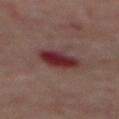Notes:
– workup — imaged on a skin check; not biopsied
– location — the mid back
– imaging modality — ~15 mm tile from a whole-body skin photo
– lesion diameter — about 4.5 mm
– patient — male, in their 70s
– tile lighting — cross-polarized
– TBP lesion metrics — a border-irregularity rating of about 2/10, internal color variation of about 5.5 on a 0–10 scale, and radial color variation of about 2; a nevus-likeness score of about 0/100